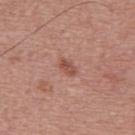notes = imaged on a skin check; not biopsied | size = ≈2.5 mm | automated lesion analysis = an average lesion color of about L≈51 a*≈24 b*≈27 (CIELAB), roughly 9 lightness units darker than nearby skin, and a normalized lesion–skin contrast near 6.5 | anatomic site = the upper back | imaging modality = ~15 mm crop, total-body skin-cancer survey | patient = male, about 40 years old | illumination = white-light.